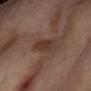Part of a total-body skin-imaging series; this lesion was reviewed on a skin check and was not flagged for biopsy.
A female subject aged approximately 55.
This is a cross-polarized tile.
About 4 mm across.
Cropped from a whole-body photographic skin survey; the tile spans about 15 mm.
Located on the abdomen.